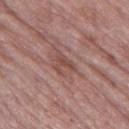Part of a total-body skin-imaging series; this lesion was reviewed on a skin check and was not flagged for biopsy.
A female subject, roughly 85 years of age or older.
A 15 mm close-up extracted from a 3D total-body photography capture.
Captured under white-light illumination.
The lesion is on the left thigh.
About 3 mm across.